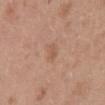| feature | finding |
|---|---|
| workup | total-body-photography surveillance lesion; no biopsy |
| tile lighting | white-light |
| location | the front of the torso |
| lesion diameter | ≈2.5 mm |
| image-analysis metrics | a footprint of about 3.5 mm² and an eccentricity of roughly 0.75; border irregularity of about 2.5 on a 0–10 scale and a color-variation rating of about 2/10; a classifier nevus-likeness of about 0/100 and lesion-presence confidence of about 100/100 |
| patient | female, aged 38 to 42 |
| image source | total-body-photography crop, ~15 mm field of view |